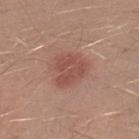About 4 mm across.
A close-up tile cropped from a whole-body skin photograph, about 15 mm across.
An algorithmic analysis of the crop reported a footprint of about 10 mm², an outline eccentricity of about 0.5 (0 = round, 1 = elongated), and a shape-asymmetry score of about 0.25 (0 = symmetric). It also reported an average lesion color of about L≈50 a*≈24 b*≈26 (CIELAB), a lesion–skin lightness drop of about 9, and a normalized border contrast of about 6.5. The software also gave a nevus-likeness score of about 90/100 and a lesion-detection confidence of about 100/100.
The lesion is located on the left lower leg.
The tile uses white-light illumination.
A male patient, approximately 30 years of age.